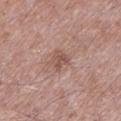| feature | finding |
|---|---|
| biopsy status | imaged on a skin check; not biopsied |
| lesion diameter | ≈3 mm |
| image source | ~15 mm tile from a whole-body skin photo |
| site | the left lower leg |
| illumination | white-light illumination |
| subject | male, aged 53–57 |